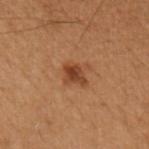The lesion-visualizer software estimated a footprint of about 4 mm², an outline eccentricity of about 0.7 (0 = round, 1 = elongated), and a symmetry-axis asymmetry near 0.35. And it measured a border-irregularity index near 3.5/10, internal color variation of about 2.5 on a 0–10 scale, and radial color variation of about 1. The analysis additionally found lesion-presence confidence of about 100/100.
A 15 mm close-up tile from a total-body photography series done for melanoma screening.
Imaged with cross-polarized lighting.
The lesion is located on the arm.
A female subject, aged approximately 50.